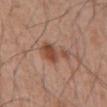A roughly 15 mm field-of-view crop from a total-body skin photograph. A male subject approximately 55 years of age. Located on the front of the torso. Captured under white-light illumination.